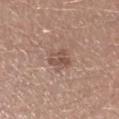Image and clinical context:
Measured at roughly 3 mm in maximum diameter. The total-body-photography lesion software estimated a footprint of about 5.5 mm², an outline eccentricity of about 0.75 (0 = round, 1 = elongated), and a symmetry-axis asymmetry near 0.3. The analysis additionally found a lesion–skin lightness drop of about 9 and a normalized lesion–skin contrast near 6.5. The subject is a male aged 28–32. A lesion tile, about 15 mm wide, cut from a 3D total-body photograph. Captured under white-light illumination.